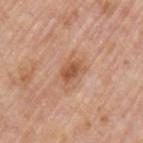biopsy_status: not biopsied; imaged during a skin examination
image:
  source: total-body photography crop
  field_of_view_mm: 15
lighting: white-light
patient:
  sex: male
  age_approx: 75
site: left upper arm
automated_metrics:
  nevus_likeness_0_100: 60
  lesion_detection_confidence_0_100: 100
lesion_size:
  long_diameter_mm_approx: 3.0